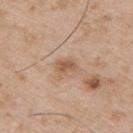{
  "biopsy_status": "not biopsied; imaged during a skin examination",
  "patient": {
    "sex": "male",
    "age_approx": 55
  },
  "image": {
    "source": "total-body photography crop",
    "field_of_view_mm": 15
  },
  "automated_metrics": {
    "nevus_likeness_0_100": 0,
    "lesion_detection_confidence_0_100": 100
  },
  "lighting": "white-light",
  "site": "upper back",
  "lesion_size": {
    "long_diameter_mm_approx": 2.5
  }
}